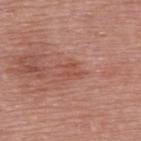Captured during whole-body skin photography for melanoma surveillance; the lesion was not biopsied.
About 3 mm across.
An algorithmic analysis of the crop reported an average lesion color of about L≈51 a*≈25 b*≈30 (CIELAB), about 6 CIELAB-L* units darker than the surrounding skin, and a lesion-to-skin contrast of about 5.5 (normalized; higher = more distinct). And it measured an automated nevus-likeness rating near 0 out of 100 and a lesion-detection confidence of about 100/100.
A region of skin cropped from a whole-body photographic capture, roughly 15 mm wide.
Imaged with white-light lighting.
A female patient, roughly 65 years of age.
The lesion is located on the chest.